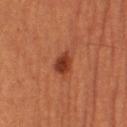| feature | finding |
|---|---|
| workup | imaged on a skin check; not biopsied |